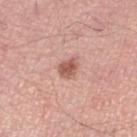{"biopsy_status": "not biopsied; imaged during a skin examination", "site": "leg", "lesion_size": {"long_diameter_mm_approx": 2.5}, "image": {"source": "total-body photography crop", "field_of_view_mm": 15}, "automated_metrics": {"border_irregularity_0_10": 2.0, "color_variation_0_10": 2.5, "peripheral_color_asymmetry": 1.0, "nevus_likeness_0_100": 90}, "lighting": "white-light", "patient": {"sex": "male", "age_approx": 70}}